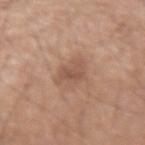A male subject, aged around 60. A lesion tile, about 15 mm wide, cut from a 3D total-body photograph. The lesion is located on the arm. Longest diameter approximately 3 mm. The tile uses white-light illumination.